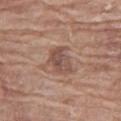Recorded during total-body skin imaging; not selected for excision or biopsy. This is a white-light tile. On the right thigh. A female patient, aged around 75. A 15 mm close-up extracted from a 3D total-body photography capture. Approximately 3.5 mm at its widest.